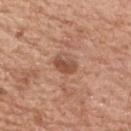<lesion>
  <biopsy_status>not biopsied; imaged during a skin examination</biopsy_status>
  <patient>
    <sex>male</sex>
    <age_approx>70</age_approx>
  </patient>
  <automated_metrics>
    <area_mm2_approx>4.0</area_mm2_approx>
    <eccentricity>0.8</eccentricity>
    <shape_asymmetry>0.3</shape_asymmetry>
    <border_irregularity_0_10>2.5</border_irregularity_0_10>
    <color_variation_0_10>3.5</color_variation_0_10>
    <peripheral_color_asymmetry>1.0</peripheral_color_asymmetry>
  </automated_metrics>
  <lighting>white-light</lighting>
  <lesion_size>
    <long_diameter_mm_approx>3.0</long_diameter_mm_approx>
  </lesion_size>
  <site>front of the torso</site>
  <image>
    <source>total-body photography crop</source>
    <field_of_view_mm>15</field_of_view_mm>
  </image>
</lesion>